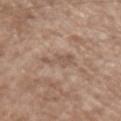The lesion is on the left forearm. Longest diameter approximately 3 mm. This is a white-light tile. The lesion-visualizer software estimated a footprint of about 4 mm², a shape eccentricity near 0.85, and a symmetry-axis asymmetry near 0.55. The analysis additionally found a classifier nevus-likeness of about 0/100 and a detector confidence of about 95 out of 100 that the crop contains a lesion. The subject is a male in their mid- to late 50s. A close-up tile cropped from a whole-body skin photograph, about 15 mm across.